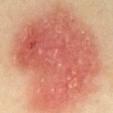  biopsy_status: not biopsied; imaged during a skin examination
  site: chest
  lighting: cross-polarized
  image:
    source: total-body photography crop
    field_of_view_mm: 15
  patient:
    sex: female
    age_approx: 35